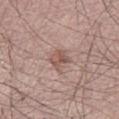image-analysis metrics — an area of roughly 4.5 mm² and a shape-asymmetry score of about 0.35 (0 = symmetric); border irregularity of about 4 on a 0–10 scale and a color-variation rating of about 4/10; lesion-presence confidence of about 100/100
tile lighting — white-light
image — ~15 mm crop, total-body skin-cancer survey
lesion diameter — ≈3 mm
patient — male, in their mid-50s